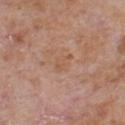Part of a total-body skin-imaging series; this lesion was reviewed on a skin check and was not flagged for biopsy. A male patient, about 65 years old. Automated tile analysis of the lesion measured a footprint of about 3.5 mm² and a shape-asymmetry score of about 0.5 (0 = symmetric). It also reported a mean CIELAB color near L≈55 a*≈21 b*≈32 and a normalized lesion–skin contrast near 4.5. The analysis additionally found border irregularity of about 5 on a 0–10 scale, a color-variation rating of about 1.5/10, and a peripheral color-asymmetry measure near 0.5. And it measured an automated nevus-likeness rating near 0 out of 100 and a detector confidence of about 100 out of 100 that the crop contains a lesion. A roughly 15 mm field-of-view crop from a total-body skin photograph. The recorded lesion diameter is about 2.5 mm. Located on the chest. Captured under white-light illumination.